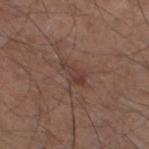biopsy status: total-body-photography surveillance lesion; no biopsy | subject: male, aged approximately 45 | image-analysis metrics: a lesion area of about 4 mm² and an outline eccentricity of about 0.9 (0 = round, 1 = elongated); a lesion color around L≈38 a*≈18 b*≈23 in CIELAB, about 7 CIELAB-L* units darker than the surrounding skin, and a normalized lesion–skin contrast near 6 | location: the left lower leg | acquisition: total-body-photography crop, ~15 mm field of view.